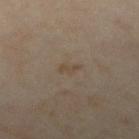Q: Was a biopsy performed?
A: catalogued during a skin exam; not biopsied
Q: Who is the patient?
A: female, in their mid- to late 30s
Q: Where on the body is the lesion?
A: the leg
Q: What kind of image is this?
A: total-body-photography crop, ~15 mm field of view